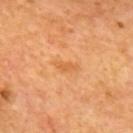{"biopsy_status": "not biopsied; imaged during a skin examination", "image": {"source": "total-body photography crop", "field_of_view_mm": 15}, "patient": {"sex": "male", "age_approx": 65}, "automated_metrics": {"border_irregularity_0_10": 4.0, "nevus_likeness_0_100": 0, "lesion_detection_confidence_0_100": 100}, "lighting": "cross-polarized", "lesion_size": {"long_diameter_mm_approx": 2.5}, "site": "upper back"}